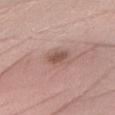workup = no biopsy performed (imaged during a skin exam) | patient = female, about 55 years old | illumination = white-light illumination | diameter = ≈3 mm | automated lesion analysis = a border-irregularity rating of about 2/10, a color-variation rating of about 1.5/10, and peripheral color asymmetry of about 0.5; a lesion-detection confidence of about 100/100 | site = the left forearm | image source = ~15 mm crop, total-body skin-cancer survey.